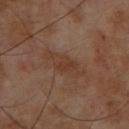<tbp_lesion>
<biopsy_status>not biopsied; imaged during a skin examination</biopsy_status>
<site>chest</site>
<patient>
  <sex>male</sex>
  <age_approx>60</age_approx>
</patient>
<lesion_size>
  <long_diameter_mm_approx>5.5</long_diameter_mm_approx>
</lesion_size>
<automated_metrics>
  <area_mm2_approx>9.5</area_mm2_approx>
  <shape_asymmetry>0.2</shape_asymmetry>
  <cielab_L>34</cielab_L>
  <cielab_a>16</cielab_a>
  <cielab_b>25</cielab_b>
  <vs_skin_darker_L>5.0</vs_skin_darker_L>
  <vs_skin_contrast_norm>5.5</vs_skin_contrast_norm>
  <lesion_detection_confidence_0_100>100</lesion_detection_confidence_0_100>
</automated_metrics>
<image>
  <source>total-body photography crop</source>
  <field_of_view_mm>15</field_of_view_mm>
</image>
</tbp_lesion>